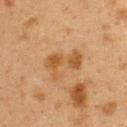follow-up — imaged on a skin check; not biopsied | lighting — cross-polarized | anatomic site — the back | image — ~15 mm crop, total-body skin-cancer survey | diameter — about 4.5 mm | subject — female, in their 40s.